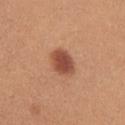{
  "biopsy_status": "not biopsied; imaged during a skin examination",
  "image": {
    "source": "total-body photography crop",
    "field_of_view_mm": 15
  },
  "site": "right upper arm",
  "lighting": "white-light",
  "lesion_size": {
    "long_diameter_mm_approx": 3.5
  },
  "patient": {
    "sex": "female",
    "age_approx": 25
  }
}